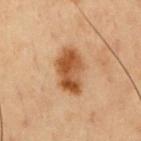* notes — total-body-photography surveillance lesion; no biopsy
* anatomic site — the chest
* image — ~15 mm crop, total-body skin-cancer survey
* lesion diameter — ~5 mm (longest diameter)
* automated metrics — an average lesion color of about L≈42 a*≈20 b*≈33 (CIELAB), about 13 CIELAB-L* units darker than the surrounding skin, and a normalized border contrast of about 10.5; border irregularity of about 2.5 on a 0–10 scale and a peripheral color-asymmetry measure near 2; a classifier nevus-likeness of about 95/100 and lesion-presence confidence of about 100/100
* patient — male, aged 53–57
* tile lighting — cross-polarized